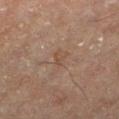– notes · total-body-photography surveillance lesion; no biopsy
– tile lighting · cross-polarized
– subject · male, roughly 65 years of age
– location · the left lower leg
– image source · total-body-photography crop, ~15 mm field of view